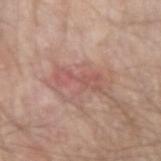Part of a total-body skin-imaging series; this lesion was reviewed on a skin check and was not flagged for biopsy. The recorded lesion diameter is about 5.5 mm. The lesion-visualizer software estimated a lesion area of about 8 mm² and a shape-asymmetry score of about 0.45 (0 = symmetric). It also reported an average lesion color of about L≈55 a*≈23 b*≈25 (CIELAB), a lesion–skin lightness drop of about 8, and a lesion-to-skin contrast of about 5.5 (normalized; higher = more distinct). The analysis additionally found a color-variation rating of about 3/10 and peripheral color asymmetry of about 1. Located on the right forearm. A 15 mm close-up extracted from a 3D total-body photography capture. Imaged with white-light lighting. A male patient, in their mid-60s.